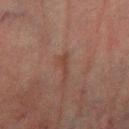Case summary:
- notes: imaged on a skin check; not biopsied
- patient: male, roughly 75 years of age
- body site: the right lower leg
- imaging modality: 15 mm crop, total-body photography